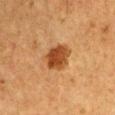| field | value |
|---|---|
| notes | catalogued during a skin exam; not biopsied |
| lesion diameter | ~4 mm (longest diameter) |
| location | the left upper arm |
| lighting | cross-polarized |
| imaging modality | ~15 mm tile from a whole-body skin photo |
| subject | male, aged 48 to 52 |
| TBP lesion metrics | an area of roughly 9.5 mm² and a symmetry-axis asymmetry near 0.15; a border-irregularity rating of about 1.5/10 |